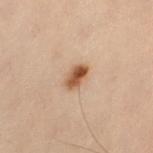{"biopsy_status": "not biopsied; imaged during a skin examination", "lighting": "cross-polarized", "site": "abdomen", "patient": {"sex": "female", "age_approx": 60}, "lesion_size": {"long_diameter_mm_approx": 3.0}, "image": {"source": "total-body photography crop", "field_of_view_mm": 15}}